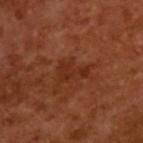notes: catalogued during a skin exam; not biopsied | tile lighting: cross-polarized illumination | TBP lesion metrics: an area of roughly 5 mm² and an outline eccentricity of about 0.85 (0 = round, 1 = elongated); an automated nevus-likeness rating near 0 out of 100 and lesion-presence confidence of about 100/100 | imaging modality: total-body-photography crop, ~15 mm field of view | patient: male, about 65 years old.